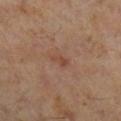Part of a total-body skin-imaging series; this lesion was reviewed on a skin check and was not flagged for biopsy.
A male subject aged around 60.
A close-up tile cropped from a whole-body skin photograph, about 15 mm across.
Captured under cross-polarized illumination.
The lesion is on the right lower leg.